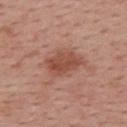Case summary:
• biopsy status — imaged on a skin check; not biopsied
• image-analysis metrics — a footprint of about 11 mm²; an automated nevus-likeness rating near 75 out of 100 and a lesion-detection confidence of about 100/100
• patient — female, about 55 years old
• tile lighting — white-light illumination
• body site — the upper back
• lesion diameter — ≈4.5 mm
• acquisition — ~15 mm crop, total-body skin-cancer survey